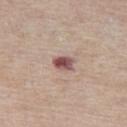Notes:
- follow-up: total-body-photography surveillance lesion; no biopsy
- illumination: white-light illumination
- lesion diameter: about 2.5 mm
- patient: female, approximately 60 years of age
- site: the left thigh
- image: total-body-photography crop, ~15 mm field of view